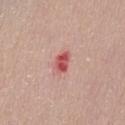biopsy_status: not biopsied; imaged during a skin examination
site: abdomen
patient:
  sex: female
  age_approx: 80
image:
  source: total-body photography crop
  field_of_view_mm: 15